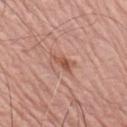diameter: ≈3 mm
patient: male, aged approximately 75
image-analysis metrics: a lesion area of about 4 mm² and a symmetry-axis asymmetry near 0.4; a border-irregularity rating of about 4/10, a within-lesion color-variation index near 2/10, and peripheral color asymmetry of about 1
acquisition: 15 mm crop, total-body photography
site: the right upper arm
illumination: white-light